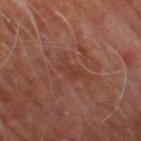| field | value |
|---|---|
| biopsy status | no biopsy performed (imaged during a skin exam) |
| acquisition | ~15 mm tile from a whole-body skin photo |
| anatomic site | the right thigh |
| lesion size | ~3 mm (longest diameter) |
| automated metrics | an average lesion color of about L≈30 a*≈20 b*≈23 (CIELAB), a lesion–skin lightness drop of about 4, and a normalized border contrast of about 4.5; peripheral color asymmetry of about 0 |
| subject | male, aged around 70 |